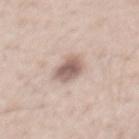The lesion was tiled from a total-body skin photograph and was not biopsied. The lesion's longest dimension is about 4 mm. A 15 mm crop from a total-body photograph taken for skin-cancer surveillance. From the mid back. The tile uses white-light illumination. A male subject aged 38–42. Automated image analysis of the tile measured a footprint of about 7.5 mm².